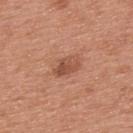Clinical impression: This lesion was catalogued during total-body skin photography and was not selected for biopsy. Context: A male subject, aged around 55. The lesion is located on the upper back. Cropped from a total-body skin-imaging series; the visible field is about 15 mm. Imaged with white-light lighting.